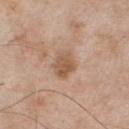<lesion>
<biopsy_status>not biopsied; imaged during a skin examination</biopsy_status>
<automated_metrics>
  <cielab_L>55</cielab_L>
  <cielab_a>19</cielab_a>
  <cielab_b>31</cielab_b>
  <vs_skin_contrast_norm>7.0</vs_skin_contrast_norm>
</automated_metrics>
<lesion_size>
  <long_diameter_mm_approx>3.0</long_diameter_mm_approx>
</lesion_size>
<patient>
  <sex>male</sex>
  <age_approx>55</age_approx>
</patient>
<image>
  <source>total-body photography crop</source>
  <field_of_view_mm>15</field_of_view_mm>
</image>
<lighting>white-light</lighting>
<site>chest</site>
</lesion>